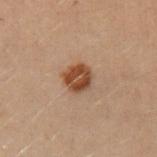Q: Was this lesion biopsied?
A: imaged on a skin check; not biopsied
Q: What is the anatomic site?
A: the left forearm
Q: What are the patient's age and sex?
A: female, in their 30s
Q: How large is the lesion?
A: about 3 mm
Q: What did automated image analysis measure?
A: a footprint of about 7.5 mm², a shape eccentricity near 0.25, and two-axis asymmetry of about 0.2; a mean CIELAB color near L≈37 a*≈18 b*≈27, about 11 CIELAB-L* units darker than the surrounding skin, and a normalized border contrast of about 10; a border-irregularity index near 2/10, internal color variation of about 4 on a 0–10 scale, and radial color variation of about 1.5
Q: What kind of image is this?
A: ~15 mm crop, total-body skin-cancer survey